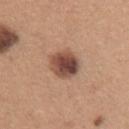This lesion was catalogued during total-body skin photography and was not selected for biopsy. The lesion-visualizer software estimated internal color variation of about 7.5 on a 0–10 scale and a peripheral color-asymmetry measure near 2.5. The analysis additionally found an automated nevus-likeness rating near 90 out of 100 and lesion-presence confidence of about 100/100. A region of skin cropped from a whole-body photographic capture, roughly 15 mm wide. A female subject, about 30 years old. The lesion is located on the upper back. Captured under white-light illumination. About 4 mm across.